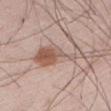Recorded during total-body skin imaging; not selected for excision or biopsy.
The lesion is located on the abdomen.
Automated tile analysis of the lesion measured a footprint of about 12 mm², an outline eccentricity of about 0.95 (0 = round, 1 = elongated), and two-axis asymmetry of about 0.6. The software also gave a border-irregularity index near 8.5/10 and a within-lesion color-variation index near 5/10. The analysis additionally found an automated nevus-likeness rating near 100 out of 100 and lesion-presence confidence of about 100/100.
A 15 mm crop from a total-body photograph taken for skin-cancer surveillance.
The subject is a male aged 58–62.
The tile uses white-light illumination.